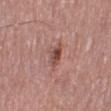Recorded during total-body skin imaging; not selected for excision or biopsy. The lesion is on the left lower leg. The lesion-visualizer software estimated a lesion area of about 4.5 mm² and a shape-asymmetry score of about 0.3 (0 = symmetric). The software also gave a lesion color around L≈48 a*≈23 b*≈25 in CIELAB, roughly 11 lightness units darker than nearby skin, and a normalized lesion–skin contrast near 8. It also reported a classifier nevus-likeness of about 25/100 and a lesion-detection confidence of about 100/100. A 15 mm close-up extracted from a 3D total-body photography capture. The tile uses white-light illumination. A male subject in their mid- to late 70s.